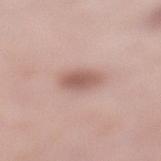About 3.5 mm across. Imaged with white-light lighting. Cropped from a whole-body photographic skin survey; the tile spans about 15 mm. The lesion is located on the left lower leg. A female patient in their 40s.